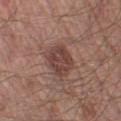{"biopsy_status": "not biopsied; imaged during a skin examination", "lighting": "white-light", "image": {"source": "total-body photography crop", "field_of_view_mm": 15}, "patient": {"sex": "male", "age_approx": 55}, "site": "left thigh"}